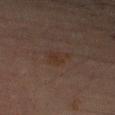Q: How was the tile lit?
A: cross-polarized
Q: What kind of image is this?
A: 15 mm crop, total-body photography
Q: How large is the lesion?
A: about 3 mm
Q: What are the patient's age and sex?
A: male, aged approximately 70
Q: Lesion location?
A: the right upper arm